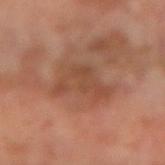Clinical impression:
Recorded during total-body skin imaging; not selected for excision or biopsy.
Background:
From the left forearm. A male patient in their 50s. A lesion tile, about 15 mm wide, cut from a 3D total-body photograph.